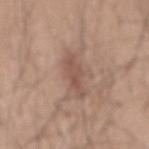- notes: catalogued during a skin exam; not biopsied
- subject: male, roughly 45 years of age
- anatomic site: the mid back
- acquisition: ~15 mm tile from a whole-body skin photo